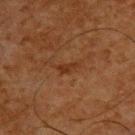Clinical impression:
Part of a total-body skin-imaging series; this lesion was reviewed on a skin check and was not flagged for biopsy.